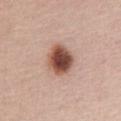Q: Was this lesion biopsied?
A: no biopsy performed (imaged during a skin exam)
Q: What is the lesion's diameter?
A: about 4 mm
Q: What kind of image is this?
A: 15 mm crop, total-body photography
Q: What did automated image analysis measure?
A: a footprint of about 11 mm² and two-axis asymmetry of about 0.1
Q: What lighting was used for the tile?
A: white-light
Q: What are the patient's age and sex?
A: female, roughly 60 years of age
Q: Lesion location?
A: the chest